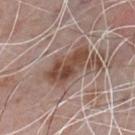| field | value |
|---|---|
| notes | no biopsy performed (imaged during a skin exam) |
| site | the chest |
| image | ~15 mm tile from a whole-body skin photo |
| subject | male, approximately 70 years of age |
| tile lighting | white-light illumination |
| lesion diameter | about 5.5 mm |